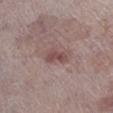{
  "biopsy_status": "not biopsied; imaged during a skin examination",
  "lesion_size": {
    "long_diameter_mm_approx": 3.0
  },
  "lighting": "white-light",
  "patient": {
    "sex": "male",
    "age_approx": 80
  },
  "site": "right lower leg",
  "automated_metrics": {
    "border_irregularity_0_10": 3.5,
    "color_variation_0_10": 2.0,
    "peripheral_color_asymmetry": 1.0
  },
  "image": {
    "source": "total-body photography crop",
    "field_of_view_mm": 15
  }
}